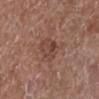Background: The lesion is on the leg. The subject is a female roughly 75 years of age. Cropped from a total-body skin-imaging series; the visible field is about 15 mm.